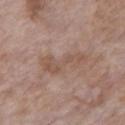Q: Was a biopsy performed?
A: no biopsy performed (imaged during a skin exam)
Q: Who is the patient?
A: female, aged approximately 85 or older
Q: How was the tile lit?
A: white-light illumination
Q: How large is the lesion?
A: ≈6 mm
Q: What is the imaging modality?
A: 15 mm crop, total-body photography
Q: Where on the body is the lesion?
A: the chest
Q: What did automated image analysis measure?
A: a mean CIELAB color near L≈53 a*≈17 b*≈26 and a lesion–skin lightness drop of about 7